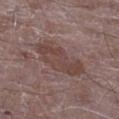Notes:
– notes: total-body-photography surveillance lesion; no biopsy
– location: the left thigh
– imaging modality: 15 mm crop, total-body photography
– subject: male, aged around 65
– diameter: ~6.5 mm (longest diameter)
– TBP lesion metrics: an area of roughly 15 mm² and a shape-asymmetry score of about 0.25 (0 = symmetric); a classifier nevus-likeness of about 0/100 and a lesion-detection confidence of about 100/100